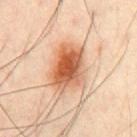  biopsy_status: not biopsied; imaged during a skin examination
  patient:
    sex: male
    age_approx: 45
  automated_metrics:
    border_irregularity_0_10: 2.5
    color_variation_0_10: 9.0
    peripheral_color_asymmetry: 3.0
    nevus_likeness_0_100: 100
    lesion_detection_confidence_0_100: 100
  image:
    source: total-body photography crop
    field_of_view_mm: 15
  site: chest
  lighting: cross-polarized